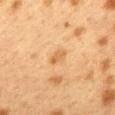Recorded during total-body skin imaging; not selected for excision or biopsy.
Cropped from a whole-body photographic skin survey; the tile spans about 15 mm.
Imaged with cross-polarized lighting.
The lesion is on the mid back.
A female subject aged 38–42.
The lesion's longest dimension is about 2.5 mm.